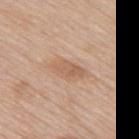Automated image analysis of the tile measured an area of roughly 5 mm² and two-axis asymmetry of about 0.25. The analysis additionally found a mean CIELAB color near L≈59 a*≈19 b*≈32. A roughly 15 mm field-of-view crop from a total-body skin photograph. A male patient, in their 80s. Imaged with white-light lighting. Approximately 3.5 mm at its widest. Located on the upper back.